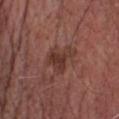Clinical impression:
Imaged during a routine full-body skin examination; the lesion was not biopsied and no histopathology is available.
Clinical summary:
A male patient, approximately 65 years of age. Measured at roughly 3.5 mm in maximum diameter. Cropped from a whole-body photographic skin survey; the tile spans about 15 mm. From the front of the torso. The tile uses white-light illumination.